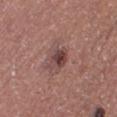follow-up = imaged on a skin check; not biopsied | imaging modality = ~15 mm crop, total-body skin-cancer survey | site = the leg | diameter = ≈3.5 mm | lighting = white-light illumination | patient = female, aged approximately 40 | image-analysis metrics = a border-irregularity rating of about 2/10, a color-variation rating of about 5/10, and radial color variation of about 1.5.